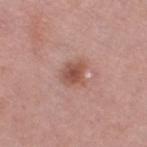biopsy status: no biopsy performed (imaged during a skin exam)
lesion size: ≈3 mm
patient: female, about 70 years old
imaging modality: ~15 mm tile from a whole-body skin photo
location: the leg